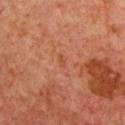{
  "biopsy_status": "not biopsied; imaged during a skin examination",
  "lighting": "cross-polarized",
  "site": "chest",
  "lesion_size": {
    "long_diameter_mm_approx": 1.0
  },
  "patient": {
    "sex": "female",
    "age_approx": 40
  },
  "image": {
    "source": "total-body photography crop",
    "field_of_view_mm": 15
  }
}